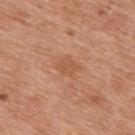Background: On the upper back. About 2.5 mm across. The tile uses white-light illumination. The total-body-photography lesion software estimated a lesion–skin lightness drop of about 6 and a normalized border contrast of about 5. Cropped from a total-body skin-imaging series; the visible field is about 15 mm. The patient is a male approximately 70 years of age.